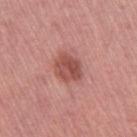Clinical impression:
This lesion was catalogued during total-body skin photography and was not selected for biopsy.
Image and clinical context:
A female patient aged approximately 65. A lesion tile, about 15 mm wide, cut from a 3D total-body photograph. The lesion is located on the left thigh.